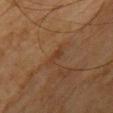Impression: Recorded during total-body skin imaging; not selected for excision or biopsy. Context: From the chest. Imaged with cross-polarized lighting. The lesion's longest dimension is about 3 mm. A female subject, aged around 55. A lesion tile, about 15 mm wide, cut from a 3D total-body photograph. The total-body-photography lesion software estimated a footprint of about 3 mm², an outline eccentricity of about 0.9 (0 = round, 1 = elongated), and a shape-asymmetry score of about 0.35 (0 = symmetric). The analysis additionally found a border-irregularity index near 3.5/10, internal color variation of about 0.5 on a 0–10 scale, and peripheral color asymmetry of about 0. The analysis additionally found a classifier nevus-likeness of about 0/100 and lesion-presence confidence of about 100/100.